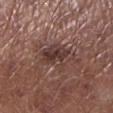Q: Was a biopsy performed?
A: imaged on a skin check; not biopsied
Q: Automated lesion metrics?
A: an area of roughly 11 mm², an eccentricity of roughly 0.7, and a shape-asymmetry score of about 0.3 (0 = symmetric); an average lesion color of about L≈37 a*≈18 b*≈20 (CIELAB), about 9 CIELAB-L* units darker than the surrounding skin, and a normalized lesion–skin contrast near 8; a classifier nevus-likeness of about 0/100
Q: Patient demographics?
A: male, aged approximately 55
Q: How was the tile lit?
A: white-light illumination
Q: What is the anatomic site?
A: the leg
Q: What kind of image is this?
A: ~15 mm tile from a whole-body skin photo
Q: Lesion size?
A: ≈4.5 mm